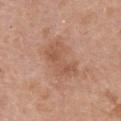Captured during whole-body skin photography for melanoma surveillance; the lesion was not biopsied. This image is a 15 mm lesion crop taken from a total-body photograph. The lesion is on the chest. A female subject, roughly 60 years of age. The lesion's longest dimension is about 5 mm.